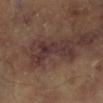– notes: imaged on a skin check; not biopsied
– lighting: cross-polarized
– image: 15 mm crop, total-body photography
– TBP lesion metrics: a shape eccentricity near 0.85 and a shape-asymmetry score of about 0.7 (0 = symmetric); an average lesion color of about L≈32 a*≈18 b*≈17 (CIELAB), roughly 8 lightness units darker than nearby skin, and a normalized lesion–skin contrast near 9; a border-irregularity index near 9/10, a color-variation rating of about 4/10, and radial color variation of about 1.5; a classifier nevus-likeness of about 0/100
– lesion size: ≈5.5 mm
– subject: aged 58–62
– location: the right lower leg